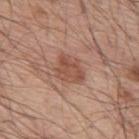| field | value |
|---|---|
| notes | imaged on a skin check; not biopsied |
| lesion diameter | about 3.5 mm |
| body site | the upper back |
| acquisition | ~15 mm tile from a whole-body skin photo |
| subject | male, aged approximately 55 |